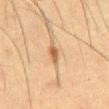Part of a total-body skin-imaging series; this lesion was reviewed on a skin check and was not flagged for biopsy. A region of skin cropped from a whole-body photographic capture, roughly 15 mm wide. From the abdomen. Captured under cross-polarized illumination. The patient is a male roughly 50 years of age. The recorded lesion diameter is about 2.5 mm.